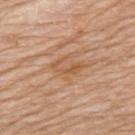Q: Was a biopsy performed?
A: total-body-photography surveillance lesion; no biopsy
Q: Who is the patient?
A: female, about 75 years old
Q: Where on the body is the lesion?
A: the upper back
Q: Lesion size?
A: ~3.5 mm (longest diameter)
Q: What lighting was used for the tile?
A: white-light illumination
Q: How was this image acquired?
A: ~15 mm crop, total-body skin-cancer survey